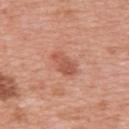Q: Was a biopsy performed?
A: total-body-photography surveillance lesion; no biopsy
Q: Lesion location?
A: the upper back
Q: Automated lesion metrics?
A: a lesion–skin lightness drop of about 10; a nevus-likeness score of about 10/100 and a detector confidence of about 100 out of 100 that the crop contains a lesion
Q: Lesion size?
A: about 3.5 mm
Q: What kind of image is this?
A: 15 mm crop, total-body photography
Q: Patient demographics?
A: female, aged 38 to 42
Q: How was the tile lit?
A: white-light illumination